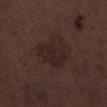Recorded during total-body skin imaging; not selected for excision or biopsy. Cropped from a total-body skin-imaging series; the visible field is about 15 mm. On the right lower leg. Captured under white-light illumination. The recorded lesion diameter is about 4.5 mm. The patient is a male aged 68 to 72.